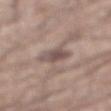Impression: Imaged during a routine full-body skin examination; the lesion was not biopsied and no histopathology is available. Clinical summary: Located on the abdomen. Captured under white-light illumination. Longest diameter approximately 4 mm. The patient is a male aged 73–77. Cropped from a total-body skin-imaging series; the visible field is about 15 mm.